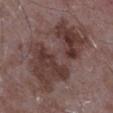<record>
<biopsy_status>not biopsied; imaged during a skin examination</biopsy_status>
<automated_metrics>
  <area_mm2_approx>41.0</area_mm2_approx>
  <shape_asymmetry>0.4</shape_asymmetry>
  <cielab_L>37</cielab_L>
  <cielab_a>18</cielab_a>
  <cielab_b>19</cielab_b>
  <vs_skin_darker_L>10.0</vs_skin_darker_L>
  <vs_skin_contrast_norm>8.5</vs_skin_contrast_norm>
  <nevus_likeness_0_100>0</nevus_likeness_0_100>
</automated_metrics>
<site>left upper arm</site>
<image>
  <source>total-body photography crop</source>
  <field_of_view_mm>15</field_of_view_mm>
</image>
<lesion_size>
  <long_diameter_mm_approx>10.5</long_diameter_mm_approx>
</lesion_size>
<patient>
  <sex>male</sex>
  <age_approx>65</age_approx>
</patient>
</record>